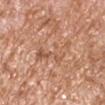| feature | finding |
|---|---|
| notes | imaged on a skin check; not biopsied |
| patient | male, aged around 65 |
| acquisition | ~15 mm tile from a whole-body skin photo |
| location | the right upper arm |